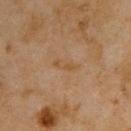{"biopsy_status": "not biopsied; imaged during a skin examination", "lighting": "cross-polarized", "patient": {"sex": "male", "age_approx": 45}, "site": "upper back", "lesion_size": {"long_diameter_mm_approx": 3.5}, "image": {"source": "total-body photography crop", "field_of_view_mm": 15}, "automated_metrics": {"cielab_L": 41, "cielab_a": 15, "cielab_b": 30, "vs_skin_contrast_norm": 5.0, "border_irregularity_0_10": 4.0, "color_variation_0_10": 0.0, "peripheral_color_asymmetry": 0.0, "nevus_likeness_0_100": 0, "lesion_detection_confidence_0_100": 100}}